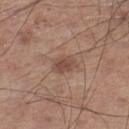This lesion was catalogued during total-body skin photography and was not selected for biopsy. The lesion's longest dimension is about 3 mm. From the left lower leg. Captured under white-light illumination. A lesion tile, about 15 mm wide, cut from a 3D total-body photograph. A male patient, aged around 60. An algorithmic analysis of the crop reported an area of roughly 4.5 mm², an outline eccentricity of about 0.7 (0 = round, 1 = elongated), and two-axis asymmetry of about 0.2. The software also gave a lesion color around L≈47 a*≈19 b*≈26 in CIELAB and a normalized border contrast of about 7. The analysis additionally found a nevus-likeness score of about 40/100 and a lesion-detection confidence of about 100/100.